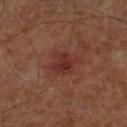Notes:
- acquisition — ~15 mm crop, total-body skin-cancer survey
- patient — male, aged 58–62
- location — the leg
- size — ~3.5 mm (longest diameter)